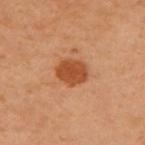{"biopsy_status": "not biopsied; imaged during a skin examination"}